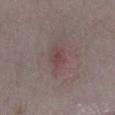The lesion was photographed on a routine skin check and not biopsied; there is no pathology result. Imaged with white-light lighting. A lesion tile, about 15 mm wide, cut from a 3D total-body photograph. The patient is a male aged approximately 75. The lesion is located on the right lower leg.